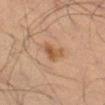follow-up: catalogued during a skin exam; not biopsied
diameter: about 3 mm
image source: 15 mm crop, total-body photography
patient: male, roughly 70 years of age
location: the leg